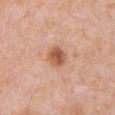{
  "biopsy_status": "not biopsied; imaged during a skin examination",
  "lighting": "white-light",
  "image": {
    "source": "total-body photography crop",
    "field_of_view_mm": 15
  },
  "site": "chest",
  "lesion_size": {
    "long_diameter_mm_approx": 3.0
  },
  "patient": {
    "sex": "male",
    "age_approx": 55
  },
  "automated_metrics": {
    "border_irregularity_0_10": 1.5,
    "color_variation_0_10": 4.5,
    "peripheral_color_asymmetry": 1.5
  }
}